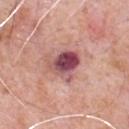biopsy_status: not biopsied; imaged during a skin examination
image:
  source: total-body photography crop
  field_of_view_mm: 15
automated_metrics:
  area_mm2_approx: 9.0
  eccentricity: 0.6
  color_variation_0_10: 10.0
  peripheral_color_asymmetry: 3.0
lesion_size:
  long_diameter_mm_approx: 4.0
site: chest
patient:
  sex: male
  age_approx: 80